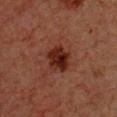imaging modality = ~15 mm crop, total-body skin-cancer survey
patient = female, in their 40s
body site = the chest